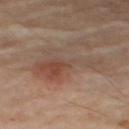Q: Is there a histopathology result?
A: no biopsy performed (imaged during a skin exam)
Q: Lesion location?
A: the right lower leg
Q: How was this image acquired?
A: ~15 mm tile from a whole-body skin photo
Q: Who is the patient?
A: female, aged approximately 65
Q: What did automated image analysis measure?
A: a mean CIELAB color near L≈48 a*≈17 b*≈26 and about 8 CIELAB-L* units darker than the surrounding skin; a classifier nevus-likeness of about 30/100 and a detector confidence of about 85 out of 100 that the crop contains a lesion
Q: What is the lesion's diameter?
A: about 9.5 mm